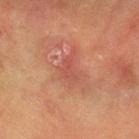workup: total-body-photography surveillance lesion; no biopsy | site: the front of the torso | subject: female, aged around 55 | automated metrics: an area of roughly 5 mm², a shape eccentricity near 0.85, and two-axis asymmetry of about 0.4; an average lesion color of about L≈46 a*≈26 b*≈27 (CIELAB), about 6 CIELAB-L* units darker than the surrounding skin, and a normalized border contrast of about 4.5; border irregularity of about 5 on a 0–10 scale and peripheral color asymmetry of about 1 | image source: 15 mm crop, total-body photography.